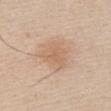Q: Was a biopsy performed?
A: no biopsy performed (imaged during a skin exam)
Q: Where on the body is the lesion?
A: the chest
Q: Automated lesion metrics?
A: an average lesion color of about L≈63 a*≈19 b*≈32 (CIELAB), about 7 CIELAB-L* units darker than the surrounding skin, and a normalized lesion–skin contrast near 5; a border-irregularity rating of about 3.5/10, a color-variation rating of about 2/10, and peripheral color asymmetry of about 0.5; an automated nevus-likeness rating near 55 out of 100
Q: Patient demographics?
A: male, aged 43 to 47
Q: Illumination type?
A: white-light
Q: What kind of image is this?
A: ~15 mm crop, total-body skin-cancer survey